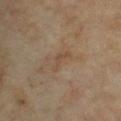workup: no biopsy performed (imaged during a skin exam); site: the chest; subject: male, about 65 years old; image: ~15 mm crop, total-body skin-cancer survey; lighting: cross-polarized.